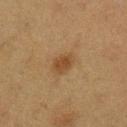This lesion was catalogued during total-body skin photography and was not selected for biopsy.
This is a cross-polarized tile.
The lesion is located on the leg.
A female patient aged 38 to 42.
A 15 mm close-up extracted from a 3D total-body photography capture.